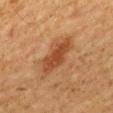| key | value |
|---|---|
| workup | catalogued during a skin exam; not biopsied |
| image | 15 mm crop, total-body photography |
| TBP lesion metrics | an area of roughly 9.5 mm², an outline eccentricity of about 0.9 (0 = round, 1 = elongated), and a shape-asymmetry score of about 0.25 (0 = symmetric); an average lesion color of about L≈39 a*≈21 b*≈32 (CIELAB) and about 9 CIELAB-L* units darker than the surrounding skin; a border-irregularity rating of about 3/10, a within-lesion color-variation index near 2.5/10, and peripheral color asymmetry of about 1; a classifier nevus-likeness of about 70/100 and a lesion-detection confidence of about 100/100 |
| body site | the mid back |
| patient | male, aged 58–62 |
| lighting | cross-polarized illumination |
| diameter | about 5 mm |